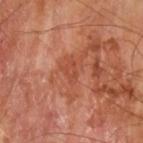Q: Lesion location?
A: the left upper arm
Q: Patient demographics?
A: male, aged 63–67
Q: How large is the lesion?
A: ≈3 mm
Q: What lighting was used for the tile?
A: cross-polarized
Q: What is the imaging modality?
A: 15 mm crop, total-body photography